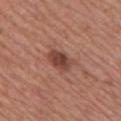Assessment: Imaged during a routine full-body skin examination; the lesion was not biopsied and no histopathology is available. Clinical summary: On the chest. The subject is a female roughly 50 years of age. A 15 mm crop from a total-body photograph taken for skin-cancer surveillance.